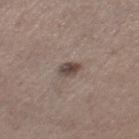The subject is a female aged approximately 65. The lesion's longest dimension is about 2.5 mm. The total-body-photography lesion software estimated a lesion area of about 4 mm², an outline eccentricity of about 0.7 (0 = round, 1 = elongated), and two-axis asymmetry of about 0.25. The software also gave a border-irregularity index near 2/10, internal color variation of about 3.5 on a 0–10 scale, and radial color variation of about 1. And it measured lesion-presence confidence of about 100/100. On the left thigh. A 15 mm close-up tile from a total-body photography series done for melanoma screening.